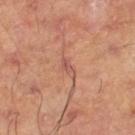Impression: Recorded during total-body skin imaging; not selected for excision or biopsy. Image and clinical context: About 3 mm across. The lesion is on the leg. A region of skin cropped from a whole-body photographic capture, roughly 15 mm wide.